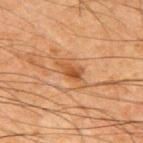Captured during whole-body skin photography for melanoma surveillance; the lesion was not biopsied.
A male subject in their mid- to late 60s.
From the upper back.
The total-body-photography lesion software estimated an area of roughly 4 mm², an outline eccentricity of about 0.85 (0 = round, 1 = elongated), and a symmetry-axis asymmetry near 0.35. It also reported a mean CIELAB color near L≈42 a*≈21 b*≈34, a lesion–skin lightness drop of about 9, and a normalized border contrast of about 7.5. The software also gave a nevus-likeness score of about 50/100 and lesion-presence confidence of about 100/100.
A roughly 15 mm field-of-view crop from a total-body skin photograph.
Imaged with cross-polarized lighting.
Approximately 3 mm at its widest.